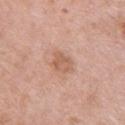Findings:
* notes — imaged on a skin check; not biopsied
* illumination — white-light
* subject — female, aged 63 to 67
* size — ≈2.5 mm
* automated lesion analysis — an area of roughly 5 mm², an eccentricity of roughly 0.6, and two-axis asymmetry of about 0.25
* site — the upper back
* imaging modality — total-body-photography crop, ~15 mm field of view